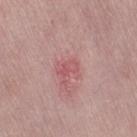{"biopsy_status": "not biopsied; imaged during a skin examination", "site": "right thigh", "patient": {"sex": "female", "age_approx": 50}, "lighting": "white-light", "image": {"source": "total-body photography crop", "field_of_view_mm": 15}, "lesion_size": {"long_diameter_mm_approx": 2.5}}